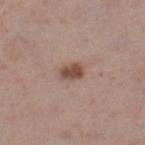Assessment:
This lesion was catalogued during total-body skin photography and was not selected for biopsy.
Background:
Captured under white-light illumination. Located on the right lower leg. The recorded lesion diameter is about 3 mm. A female subject, in their 30s. A 15 mm close-up extracted from a 3D total-body photography capture.